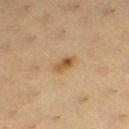Q: Was this lesion biopsied?
A: imaged on a skin check; not biopsied
Q: Automated lesion metrics?
A: a within-lesion color-variation index near 6/10 and peripheral color asymmetry of about 2; an automated nevus-likeness rating near 85 out of 100 and lesion-presence confidence of about 100/100
Q: What is the anatomic site?
A: the left lower leg
Q: How was this image acquired?
A: ~15 mm crop, total-body skin-cancer survey
Q: How was the tile lit?
A: cross-polarized illumination
Q: Who is the patient?
A: female, roughly 40 years of age